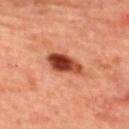The lesion was photographed on a routine skin check and not biopsied; there is no pathology result.
A close-up tile cropped from a whole-body skin photograph, about 15 mm across.
About 5 mm across.
The tile uses cross-polarized illumination.
The lesion is located on the upper back.
The subject is a female roughly 45 years of age.